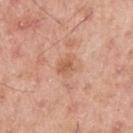Part of a total-body skin-imaging series; this lesion was reviewed on a skin check and was not flagged for biopsy. Longest diameter approximately 3 mm. A male subject, aged 53–57. A lesion tile, about 15 mm wide, cut from a 3D total-body photograph. The tile uses white-light illumination. From the arm.